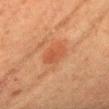Notes:
– notes · no biopsy performed (imaged during a skin exam)
– TBP lesion metrics · a footprint of about 5.5 mm² and two-axis asymmetry of about 0.2; a nevus-likeness score of about 80/100
– patient · female, roughly 55 years of age
– lesion diameter · about 3.5 mm
– lighting · cross-polarized illumination
– anatomic site · the head or neck
– image source · ~15 mm tile from a whole-body skin photo